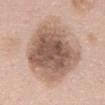<case>
  <biopsy_status>not biopsied; imaged during a skin examination</biopsy_status>
  <lighting>white-light</lighting>
  <automated_metrics>
    <vs_skin_darker_L>15.0</vs_skin_darker_L>
    <vs_skin_contrast_norm>9.5</vs_skin_contrast_norm>
    <color_variation_0_10>5.5</color_variation_0_10>
    <peripheral_color_asymmetry>1.5</peripheral_color_asymmetry>
    <nevus_likeness_0_100>5</nevus_likeness_0_100>
    <lesion_detection_confidence_0_100>100</lesion_detection_confidence_0_100>
  </automated_metrics>
  <site>chest</site>
  <image>
    <source>total-body photography crop</source>
    <field_of_view_mm>15</field_of_view_mm>
  </image>
  <lesion_size>
    <long_diameter_mm_approx>9.0</long_diameter_mm_approx>
  </lesion_size>
  <patient>
    <sex>female</sex>
    <age_approx>60</age_approx>
  </patient>
</case>